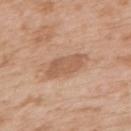Impression:
No biopsy was performed on this lesion — it was imaged during a full skin examination and was not determined to be concerning.
Acquisition and patient details:
The lesion-visualizer software estimated an area of roughly 8.5 mm², an eccentricity of roughly 0.85, and a shape-asymmetry score of about 0.25 (0 = symmetric). The software also gave an average lesion color of about L≈57 a*≈21 b*≈32 (CIELAB), a lesion–skin lightness drop of about 10, and a lesion-to-skin contrast of about 6.5 (normalized; higher = more distinct). And it measured a lesion-detection confidence of about 100/100. A female subject, aged 38 to 42. A 15 mm close-up tile from a total-body photography series done for melanoma screening. Measured at roughly 4.5 mm in maximum diameter. On the upper back.